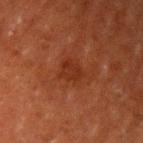Clinical impression: Captured during whole-body skin photography for melanoma surveillance; the lesion was not biopsied. Acquisition and patient details: The lesion is located on the left upper arm. Automated image analysis of the tile measured a footprint of about 5 mm², a shape eccentricity near 0.65, and two-axis asymmetry of about 0.25. The software also gave a lesion color around L≈24 a*≈23 b*≈28 in CIELAB, roughly 5 lightness units darker than nearby skin, and a lesion-to-skin contrast of about 6 (normalized; higher = more distinct). A male patient, approximately 60 years of age. The tile uses cross-polarized illumination. Longest diameter approximately 3 mm. Cropped from a whole-body photographic skin survey; the tile spans about 15 mm.